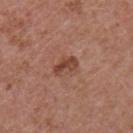Impression:
No biopsy was performed on this lesion — it was imaged during a full skin examination and was not determined to be concerning.
Clinical summary:
The recorded lesion diameter is about 3 mm. From the chest. A 15 mm crop from a total-body photograph taken for skin-cancer surveillance. A male patient, approximately 55 years of age. Imaged with white-light lighting. The lesion-visualizer software estimated a lesion area of about 4.5 mm², an outline eccentricity of about 0.8 (0 = round, 1 = elongated), and two-axis asymmetry of about 0.25. It also reported a border-irregularity rating of about 2.5/10, a within-lesion color-variation index near 4/10, and radial color variation of about 1.5. The software also gave a classifier nevus-likeness of about 25/100 and lesion-presence confidence of about 100/100.